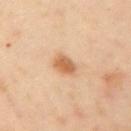No biopsy was performed on this lesion — it was imaged during a full skin examination and was not determined to be concerning.
Located on the right upper arm.
The recorded lesion diameter is about 3 mm.
A male subject, in their 40s.
This image is a 15 mm lesion crop taken from a total-body photograph.
An algorithmic analysis of the crop reported a footprint of about 5 mm² and a symmetry-axis asymmetry near 0.2.
The tile uses cross-polarized illumination.